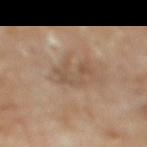The lesion is on the back.
The recorded lesion diameter is about 4.5 mm.
Captured under cross-polarized illumination.
The patient is a female aged approximately 60.
A 15 mm close-up extracted from a 3D total-body photography capture.